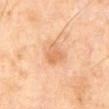Q: Was a biopsy performed?
A: no biopsy performed (imaged during a skin exam)
Q: What are the patient's age and sex?
A: male, in their mid-60s
Q: What is the anatomic site?
A: the front of the torso
Q: Illumination type?
A: cross-polarized illumination
Q: What did automated image analysis measure?
A: a lesion color around L≈64 a*≈22 b*≈38 in CIELAB, roughly 8 lightness units darker than nearby skin, and a normalized border contrast of about 5.5; a border-irregularity rating of about 2.5/10 and a peripheral color-asymmetry measure near 1; a nevus-likeness score of about 20/100
Q: How was this image acquired?
A: ~15 mm tile from a whole-body skin photo